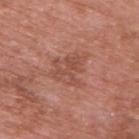| feature | finding |
|---|---|
| workup | imaged on a skin check; not biopsied |
| image source | ~15 mm tile from a whole-body skin photo |
| lesion diameter | about 4.5 mm |
| tile lighting | white-light |
| subject | male, aged 68–72 |
| body site | the upper back |
| automated metrics | a footprint of about 11 mm², a shape eccentricity near 0.5, and a shape-asymmetry score of about 0.35 (0 = symmetric); a mean CIELAB color near L≈50 a*≈25 b*≈28 and about 7 CIELAB-L* units darker than the surrounding skin; border irregularity of about 5 on a 0–10 scale and a peripheral color-asymmetry measure near 1; a classifier nevus-likeness of about 0/100 and a lesion-detection confidence of about 100/100 |